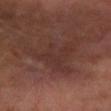| feature | finding |
|---|---|
| workup | catalogued during a skin exam; not biopsied |
| image-analysis metrics | a border-irregularity index near 8/10, a color-variation rating of about 2/10, and peripheral color asymmetry of about 0.5; an automated nevus-likeness rating near 0 out of 100 |
| acquisition | 15 mm crop, total-body photography |
| tile lighting | cross-polarized illumination |
| location | the right forearm |
| patient | male, in their mid- to late 50s |